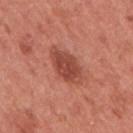{
  "biopsy_status": "not biopsied; imaged during a skin examination",
  "image": {
    "source": "total-body photography crop",
    "field_of_view_mm": 15
  },
  "lesion_size": {
    "long_diameter_mm_approx": 5.0
  },
  "lighting": "white-light",
  "site": "right upper arm",
  "patient": {
    "sex": "female",
    "age_approx": 50
  }
}